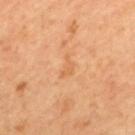Clinical impression:
Recorded during total-body skin imaging; not selected for excision or biopsy.
Image and clinical context:
The lesion's longest dimension is about 2.5 mm. A lesion tile, about 15 mm wide, cut from a 3D total-body photograph. This is a cross-polarized tile. A male patient, approximately 60 years of age. The lesion is located on the mid back.